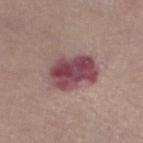Assessment:
Part of a total-body skin-imaging series; this lesion was reviewed on a skin check and was not flagged for biopsy.
Acquisition and patient details:
A close-up tile cropped from a whole-body skin photograph, about 15 mm across. The total-body-photography lesion software estimated an average lesion color of about L≈47 a*≈23 b*≈16 (CIELAB), a lesion–skin lightness drop of about 13, and a normalized lesion–skin contrast near 10.5. The software also gave a classifier nevus-likeness of about 20/100 and lesion-presence confidence of about 100/100. Longest diameter approximately 5.5 mm. Imaged with white-light lighting. The lesion is located on the leg. The patient is a male roughly 60 years of age.